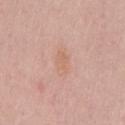Captured during whole-body skin photography for melanoma surveillance; the lesion was not biopsied. An algorithmic analysis of the crop reported a lesion area of about 4 mm², an eccentricity of roughly 0.85, and a symmetry-axis asymmetry near 0.25. The analysis additionally found a lesion color around L≈64 a*≈21 b*≈30 in CIELAB, a lesion–skin lightness drop of about 5, and a lesion-to-skin contrast of about 4.5 (normalized; higher = more distinct). It also reported a classifier nevus-likeness of about 0/100. Located on the chest. About 3 mm across. The subject is a male in their mid-60s. A 15 mm close-up tile from a total-body photography series done for melanoma screening. Imaged with white-light lighting.